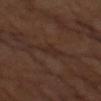The lesion was tiled from a total-body skin photograph and was not biopsied.
A male patient, in their 60s.
A 15 mm crop from a total-body photograph taken for skin-cancer surveillance.
The recorded lesion diameter is about 2.5 mm.
The lesion is on the left arm.